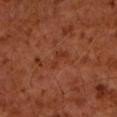<tbp_lesion>
<biopsy_status>not biopsied; imaged during a skin examination</biopsy_status>
<automated_metrics>
  <area_mm2_approx>2.5</area_mm2_approx>
  <eccentricity>0.9</eccentricity>
  <shape_asymmetry>0.55</shape_asymmetry>
  <nevus_likeness_0_100>0</nevus_likeness_0_100>
  <lesion_detection_confidence_0_100>100</lesion_detection_confidence_0_100>
</automated_metrics>
<lesion_size>
  <long_diameter_mm_approx>3.0</long_diameter_mm_approx>
</lesion_size>
<site>left forearm</site>
<image>
  <source>total-body photography crop</source>
  <field_of_view_mm>15</field_of_view_mm>
</image>
<patient>
  <sex>male</sex>
  <age_approx>60</age_approx>
</patient>
<lighting>cross-polarized</lighting>
</tbp_lesion>